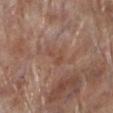Notes:
* notes: imaged on a skin check; not biopsied
* diameter: about 3 mm
* subject: male, about 70 years old
* tile lighting: white-light illumination
* imaging modality: total-body-photography crop, ~15 mm field of view
* location: the left lower leg
* automated metrics: a mean CIELAB color near L≈48 a*≈20 b*≈28, a lesion–skin lightness drop of about 6, and a lesion-to-skin contrast of about 5.5 (normalized; higher = more distinct)